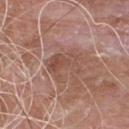Imaged during a routine full-body skin examination; the lesion was not biopsied and no histopathology is available. A male patient, aged around 55. On the chest. A lesion tile, about 15 mm wide, cut from a 3D total-body photograph. Automated tile analysis of the lesion measured a footprint of about 10 mm², a shape eccentricity near 0.75, and a symmetry-axis asymmetry near 0.45. It also reported about 7 CIELAB-L* units darker than the surrounding skin and a normalized border contrast of about 5.5. It also reported a border-irregularity rating of about 6.5/10, a color-variation rating of about 4/10, and peripheral color asymmetry of about 1.5. About 4.5 mm across. This is a white-light tile.